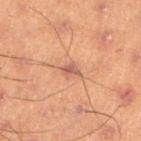{
  "biopsy_status": "not biopsied; imaged during a skin examination"
}